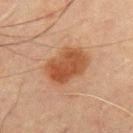subject=male, aged around 70 | diameter=~5 mm (longest diameter) | anatomic site=the front of the torso | acquisition=~15 mm tile from a whole-body skin photo | illumination=cross-polarized.